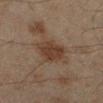patient:
  sex: male
  age_approx: 45
image:
  source: total-body photography crop
  field_of_view_mm: 15
site: left lower leg
lighting: cross-polarized
lesion_size:
  long_diameter_mm_approx: 3.5
automated_metrics:
  area_mm2_approx: 8.5
  eccentricity: 0.4
  shape_asymmetry: 0.2
  cielab_L: 30
  cielab_a: 14
  cielab_b: 22
  vs_skin_darker_L: 7.0
  vs_skin_contrast_norm: 8.0